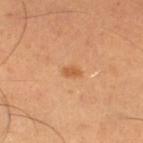Q: What is the lesion's diameter?
A: ~2 mm (longest diameter)
Q: What are the patient's age and sex?
A: female, approximately 55 years of age
Q: What kind of image is this?
A: 15 mm crop, total-body photography
Q: Lesion location?
A: the leg